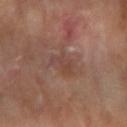Assessment:
No biopsy was performed on this lesion — it was imaged during a full skin examination and was not determined to be concerning.
Background:
The recorded lesion diameter is about 3.5 mm. The patient is a female aged approximately 60. The lesion-visualizer software estimated an area of roughly 5 mm², a shape eccentricity near 0.8, and two-axis asymmetry of about 0.45. The analysis additionally found a mean CIELAB color near L≈43 a*≈20 b*≈24, a lesion–skin lightness drop of about 6, and a normalized lesion–skin contrast near 4.5. It also reported a nevus-likeness score of about 0/100 and a lesion-detection confidence of about 95/100. The lesion is on the right forearm. A region of skin cropped from a whole-body photographic capture, roughly 15 mm wide. Imaged with cross-polarized lighting.